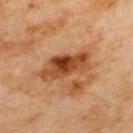biopsy status = no biopsy performed (imaged during a skin exam) | site = the upper back | imaging modality = 15 mm crop, total-body photography | diameter = ~6.5 mm (longest diameter).